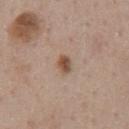Background:
On the chest. Automated image analysis of the tile measured an area of roughly 3 mm² and a shape-asymmetry score of about 0.25 (0 = symmetric). The software also gave a lesion color around L≈50 a*≈18 b*≈28 in CIELAB, about 12 CIELAB-L* units darker than the surrounding skin, and a normalized lesion–skin contrast near 9. Cropped from a whole-body photographic skin survey; the tile spans about 15 mm. The patient is a male roughly 60 years of age.